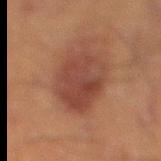No biopsy was performed on this lesion — it was imaged during a full skin examination and was not determined to be concerning.
Automated image analysis of the tile measured a symmetry-axis asymmetry near 0.15. The analysis additionally found a border-irregularity index near 2.5/10.
The tile uses cross-polarized illumination.
A male subject, approximately 45 years of age.
Cropped from a whole-body photographic skin survey; the tile spans about 15 mm.
The lesion is located on the leg.
Measured at roughly 5.5 mm in maximum diameter.